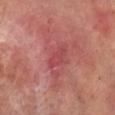Assessment:
No biopsy was performed on this lesion — it was imaged during a full skin examination and was not determined to be concerning.
Context:
The lesion's longest dimension is about 3.5 mm. On the right lower leg. Captured under cross-polarized illumination. Cropped from a whole-body photographic skin survey; the tile spans about 15 mm. The patient is a male roughly 70 years of age.